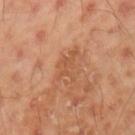Q: Was a biopsy performed?
A: catalogued during a skin exam; not biopsied
Q: What kind of image is this?
A: ~15 mm tile from a whole-body skin photo
Q: Who is the patient?
A: male, roughly 45 years of age
Q: Lesion location?
A: the left upper arm